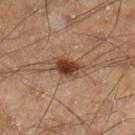This lesion was catalogued during total-body skin photography and was not selected for biopsy. Measured at roughly 4 mm in maximum diameter. A 15 mm close-up tile from a total-body photography series done for melanoma screening. An algorithmic analysis of the crop reported an eccentricity of roughly 0.75 and a shape-asymmetry score of about 0.3 (0 = symmetric). A male subject aged 48–52. Captured under cross-polarized illumination. The lesion is on the leg.